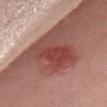The lesion was tiled from a total-body skin photograph and was not biopsied. This is a white-light tile. A female subject aged approximately 40. The total-body-photography lesion software estimated a shape eccentricity near 0.75 and two-axis asymmetry of about 0.35. And it measured an average lesion color of about L≈45 a*≈27 b*≈25 (CIELAB), a lesion–skin lightness drop of about 10, and a normalized lesion–skin contrast near 8. The analysis additionally found border irregularity of about 4.5 on a 0–10 scale, a color-variation rating of about 6/10, and radial color variation of about 2. The analysis additionally found a lesion-detection confidence of about 100/100. On the front of the torso. The recorded lesion diameter is about 6 mm. This image is a 15 mm lesion crop taken from a total-body photograph.